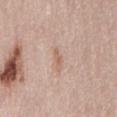Clinical impression: No biopsy was performed on this lesion — it was imaged during a full skin examination and was not determined to be concerning. Acquisition and patient details: The lesion is located on the lower back. Captured under white-light illumination. A female patient roughly 50 years of age. Cropped from a whole-body photographic skin survey; the tile spans about 15 mm. Automated tile analysis of the lesion measured a normalized lesion–skin contrast near 5.5. It also reported a border-irregularity index near 3.5/10, a within-lesion color-variation index near 0.5/10, and peripheral color asymmetry of about 0.